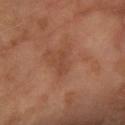Q: Was a biopsy performed?
A: total-body-photography surveillance lesion; no biopsy
Q: What is the lesion's diameter?
A: ≈2.5 mm
Q: Where on the body is the lesion?
A: the arm
Q: Automated lesion metrics?
A: a footprint of about 2 mm², an eccentricity of roughly 0.95, and a symmetry-axis asymmetry near 0.4; border irregularity of about 4.5 on a 0–10 scale, a color-variation rating of about 0/10, and a peripheral color-asymmetry measure near 0
Q: How was the tile lit?
A: cross-polarized
Q: How was this image acquired?
A: total-body-photography crop, ~15 mm field of view
Q: Who is the patient?
A: female, aged approximately 70